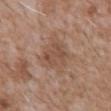On the chest.
The recorded lesion diameter is about 3.5 mm.
A lesion tile, about 15 mm wide, cut from a 3D total-body photograph.
A male subject aged around 60.